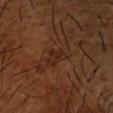biopsy status = catalogued during a skin exam; not biopsied | body site = the right forearm | imaging modality = total-body-photography crop, ~15 mm field of view | subject = male, roughly 65 years of age.